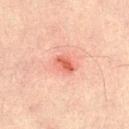Impression: This lesion was catalogued during total-body skin photography and was not selected for biopsy. Acquisition and patient details: Imaged with cross-polarized lighting. A male subject in their 30s. About 2.5 mm across. Automated image analysis of the tile measured an area of roughly 4 mm² and an eccentricity of roughly 0.8. And it measured border irregularity of about 3 on a 0–10 scale and a within-lesion color-variation index near 2/10. The software also gave a nevus-likeness score of about 35/100. On the right thigh. A lesion tile, about 15 mm wide, cut from a 3D total-body photograph.